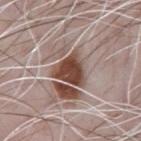Assessment: Imaged during a routine full-body skin examination; the lesion was not biopsied and no histopathology is available. Image and clinical context: The subject is a male aged around 70. About 4 mm across. The total-body-photography lesion software estimated an area of roughly 9.5 mm², an outline eccentricity of about 0.35 (0 = round, 1 = elongated), and a shape-asymmetry score of about 0.45 (0 = symmetric). And it measured a mean CIELAB color near L≈44 a*≈20 b*≈25, about 17 CIELAB-L* units darker than the surrounding skin, and a lesion-to-skin contrast of about 13 (normalized; higher = more distinct). It also reported a border-irregularity index near 4/10, a color-variation rating of about 3.5/10, and a peripheral color-asymmetry measure near 1. The analysis additionally found a classifier nevus-likeness of about 95/100 and lesion-presence confidence of about 80/100. The tile uses white-light illumination. A lesion tile, about 15 mm wide, cut from a 3D total-body photograph. The lesion is on the chest.